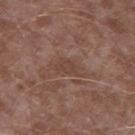<case>
<biopsy_status>not biopsied; imaged during a skin examination</biopsy_status>
<image>
  <source>total-body photography crop</source>
  <field_of_view_mm>15</field_of_view_mm>
</image>
<patient>
  <sex>male</sex>
  <age_approx>45</age_approx>
</patient>
<site>left thigh</site>
</case>